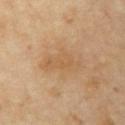biopsy status = no biopsy performed (imaged during a skin exam)
subject = female, roughly 65 years of age
image = ~15 mm tile from a whole-body skin photo
automated metrics = about 6 CIELAB-L* units darker than the surrounding skin; a border-irregularity index near 4/10, a color-variation rating of about 2/10, and a peripheral color-asymmetry measure near 0.5; an automated nevus-likeness rating near 0 out of 100 and lesion-presence confidence of about 100/100
location = the chest
lighting = cross-polarized illumination
lesion size = about 5 mm